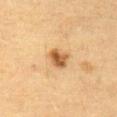Captured during whole-body skin photography for melanoma surveillance; the lesion was not biopsied. A 15 mm crop from a total-body photograph taken for skin-cancer surveillance. The recorded lesion diameter is about 2.5 mm. The tile uses cross-polarized illumination. On the front of the torso. A male subject approximately 85 years of age or older.